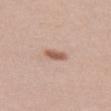<tbp_lesion>
  <biopsy_status>not biopsied; imaged during a skin examination</biopsy_status>
  <site>left thigh</site>
  <lesion_size>
    <long_diameter_mm_approx>3.0</long_diameter_mm_approx>
  </lesion_size>
  <patient>
    <sex>female</sex>
    <age_approx>20</age_approx>
  </patient>
  <image>
    <source>total-body photography crop</source>
    <field_of_view_mm>15</field_of_view_mm>
  </image>
</tbp_lesion>